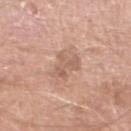Findings:
– workup · catalogued during a skin exam; not biopsied
– anatomic site · the left lower leg
– imaging modality · ~15 mm crop, total-body skin-cancer survey
– illumination · white-light
– TBP lesion metrics · a classifier nevus-likeness of about 0/100 and a detector confidence of about 100 out of 100 that the crop contains a lesion
– patient · male, approximately 80 years of age
– diameter · ≈4 mm